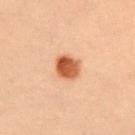Case summary:
• workup · imaged on a skin check; not biopsied
• subject · female, roughly 40 years of age
• image source · ~15 mm tile from a whole-body skin photo
• size · ~3 mm (longest diameter)
• body site · the chest
• tile lighting · cross-polarized